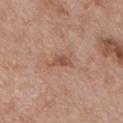Captured during whole-body skin photography for melanoma surveillance; the lesion was not biopsied. A male subject, roughly 65 years of age. About 3.5 mm across. Cropped from a whole-body photographic skin survey; the tile spans about 15 mm. From the chest. This is a white-light tile.